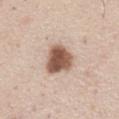Impression: The lesion was photographed on a routine skin check and not biopsied; there is no pathology result. Image and clinical context: A 15 mm close-up extracted from a 3D total-body photography capture. On the front of the torso. A male subject aged 58 to 62. Approximately 4 mm at its widest.